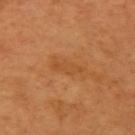Notes:
- follow-up: total-body-photography surveillance lesion; no biopsy
- anatomic site: the left upper arm
- image-analysis metrics: a lesion area of about 4.5 mm², an outline eccentricity of about 0.9 (0 = round, 1 = elongated), and a symmetry-axis asymmetry near 0.3; an automated nevus-likeness rating near 0 out of 100 and a lesion-detection confidence of about 100/100
- lesion diameter: about 3.5 mm
- acquisition: ~15 mm crop, total-body skin-cancer survey
- patient: female, in their mid- to late 50s